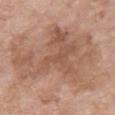The lesion is on the chest. A female patient aged 73 to 77. The lesion-visualizer software estimated an average lesion color of about L≈56 a*≈20 b*≈29 (CIELAB). A region of skin cropped from a whole-body photographic capture, roughly 15 mm wide.